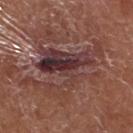Acquisition and patient details: Imaged with white-light lighting. Automated tile analysis of the lesion measured border irregularity of about 10 on a 0–10 scale, internal color variation of about 10 on a 0–10 scale, and peripheral color asymmetry of about 4. The analysis additionally found a lesion-detection confidence of about 75/100. A male subject aged 73–77. The lesion is on the left lower leg. The lesion's longest dimension is about 8 mm. A roughly 15 mm field-of-view crop from a total-body skin photograph.